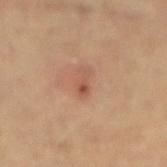This lesion was catalogued during total-body skin photography and was not selected for biopsy.
The recorded lesion diameter is about 2.5 mm.
A close-up tile cropped from a whole-body skin photograph, about 15 mm across.
Located on the arm.
A male patient aged approximately 60.
This is a cross-polarized tile.